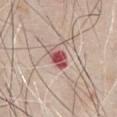Context: A male subject roughly 65 years of age. About 3 mm across. Captured under white-light illumination. The lesion is on the front of the torso. A region of skin cropped from a whole-body photographic capture, roughly 15 mm wide.